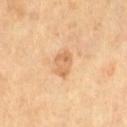notes: catalogued during a skin exam; not biopsied
acquisition: total-body-photography crop, ~15 mm field of view
diameter: about 3 mm
site: the front of the torso
subject: male, roughly 65 years of age
image-analysis metrics: an eccentricity of roughly 0.75 and a symmetry-axis asymmetry near 0.25; a lesion color around L≈64 a*≈20 b*≈38 in CIELAB, a lesion–skin lightness drop of about 9, and a normalized border contrast of about 5.5; a border-irregularity rating of about 2.5/10, a within-lesion color-variation index near 3.5/10, and peripheral color asymmetry of about 1; an automated nevus-likeness rating near 10 out of 100 and a lesion-detection confidence of about 100/100
illumination: cross-polarized